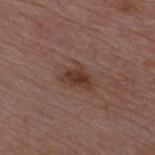| feature | finding |
|---|---|
| biopsy status | catalogued during a skin exam; not biopsied |
| acquisition | 15 mm crop, total-body photography |
| lesion size | ≈3.5 mm |
| body site | the front of the torso |
| subject | male, aged 48–52 |